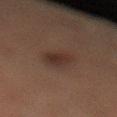Part of a total-body skin-imaging series; this lesion was reviewed on a skin check and was not flagged for biopsy. Cropped from a whole-body photographic skin survey; the tile spans about 15 mm. The lesion is located on the leg. The patient is a female in their 40s.